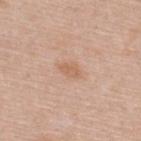A female subject aged approximately 50. This is a white-light tile. Located on the upper back. Longest diameter approximately 2.5 mm. Automated image analysis of the tile measured an average lesion color of about L≈62 a*≈20 b*≈32 (CIELAB), a lesion–skin lightness drop of about 7, and a normalized border contrast of about 5.5. The analysis additionally found a nevus-likeness score of about 5/100 and lesion-presence confidence of about 100/100. This image is a 15 mm lesion crop taken from a total-body photograph.